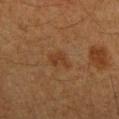workup: catalogued during a skin exam; not biopsied
image-analysis metrics: a lesion area of about 4 mm², an eccentricity of roughly 0.7, and a shape-asymmetry score of about 0.25 (0 = symmetric); a border-irregularity rating of about 3/10, internal color variation of about 2 on a 0–10 scale, and radial color variation of about 0.5; a classifier nevus-likeness of about 15/100 and a detector confidence of about 100 out of 100 that the crop contains a lesion
subject: female, aged around 40
location: the right forearm
acquisition: 15 mm crop, total-body photography